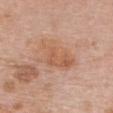Part of a total-body skin-imaging series; this lesion was reviewed on a skin check and was not flagged for biopsy. A female patient, aged around 60. Located on the chest. A 15 mm crop from a total-body photograph taken for skin-cancer surveillance. Captured under white-light illumination.